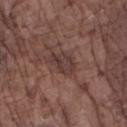The lesion was photographed on a routine skin check and not biopsied; there is no pathology result.
A male subject aged around 75.
A region of skin cropped from a whole-body photographic capture, roughly 15 mm wide.
Located on the right forearm.
The tile uses white-light illumination.
Automated image analysis of the tile measured an average lesion color of about L≈36 a*≈18 b*≈20 (CIELAB) and roughly 8 lightness units darker than nearby skin. And it measured border irregularity of about 2.5 on a 0–10 scale and a color-variation rating of about 4/10. The analysis additionally found an automated nevus-likeness rating near 0 out of 100 and lesion-presence confidence of about 90/100.